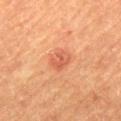workup — catalogued during a skin exam; not biopsied
illumination — cross-polarized
image — ~15 mm tile from a whole-body skin photo
anatomic site — the mid back
lesion size — ≈2.5 mm
subject — female, aged approximately 70
automated lesion analysis — a mean CIELAB color near L≈50 a*≈27 b*≈32, about 8 CIELAB-L* units darker than the surrounding skin, and a lesion-to-skin contrast of about 6 (normalized; higher = more distinct); border irregularity of about 3 on a 0–10 scale